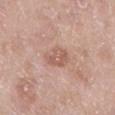biopsy_status: not biopsied; imaged during a skin examination
image:
  source: total-body photography crop
  field_of_view_mm: 15
lesion_size:
  long_diameter_mm_approx: 3.5
lighting: white-light
automated_metrics:
  area_mm2_approx: 6.0
  shape_asymmetry: 0.25
  border_irregularity_0_10: 2.5
  color_variation_0_10: 2.5
  peripheral_color_asymmetry: 1.0
  nevus_likeness_0_100: 0
  lesion_detection_confidence_0_100: 100
patient:
  sex: female
  age_approx: 70
site: left lower leg